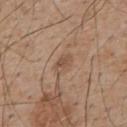Q: Is there a histopathology result?
A: catalogued during a skin exam; not biopsied
Q: Lesion location?
A: the back
Q: Illumination type?
A: white-light illumination
Q: What kind of image is this?
A: ~15 mm tile from a whole-body skin photo
Q: What did automated image analysis measure?
A: a footprint of about 4.5 mm² and two-axis asymmetry of about 0.25; a lesion color around L≈52 a*≈17 b*≈28 in CIELAB, about 7 CIELAB-L* units darker than the surrounding skin, and a normalized border contrast of about 5.5; internal color variation of about 3 on a 0–10 scale and a peripheral color-asymmetry measure near 1; a detector confidence of about 100 out of 100 that the crop contains a lesion
Q: Patient demographics?
A: male, aged around 55